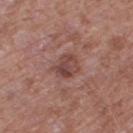Q: Was this lesion biopsied?
A: catalogued during a skin exam; not biopsied
Q: How was this image acquired?
A: 15 mm crop, total-body photography
Q: Automated lesion metrics?
A: an area of roughly 6.5 mm², a shape eccentricity near 0.6, and a shape-asymmetry score of about 0.25 (0 = symmetric); about 9 CIELAB-L* units darker than the surrounding skin and a normalized lesion–skin contrast near 7.5; a classifier nevus-likeness of about 0/100 and a lesion-detection confidence of about 100/100
Q: How was the tile lit?
A: white-light
Q: What is the anatomic site?
A: the leg
Q: Who is the patient?
A: male, in their 60s